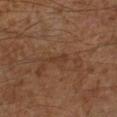Case summary:
- biopsy status · imaged on a skin check; not biopsied
- diameter · about 2.5 mm
- subject · male, aged 58 to 62
- body site · the left lower leg
- image · ~15 mm crop, total-body skin-cancer survey
- lighting · cross-polarized